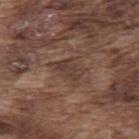workup: total-body-photography surveillance lesion; no biopsy | imaging modality: ~15 mm tile from a whole-body skin photo | subject: male, roughly 75 years of age | body site: the upper back.